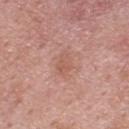Notes:
* notes: total-body-photography surveillance lesion; no biopsy
* size: ~3 mm (longest diameter)
* illumination: white-light illumination
* site: the back
* acquisition: ~15 mm crop, total-body skin-cancer survey
* subject: male, roughly 55 years of age